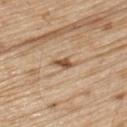<lesion>
<biopsy_status>not biopsied; imaged during a skin examination</biopsy_status>
<site>left upper arm</site>
<image>
  <source>total-body photography crop</source>
  <field_of_view_mm>15</field_of_view_mm>
</image>
<automated_metrics>
  <border_irregularity_0_10>3.5</border_irregularity_0_10>
  <color_variation_0_10>1.5</color_variation_0_10>
  <peripheral_color_asymmetry>0.5</peripheral_color_asymmetry>
  <nevus_likeness_0_100>55</nevus_likeness_0_100>
  <lesion_detection_confidence_0_100>100</lesion_detection_confidence_0_100>
</automated_metrics>
<patient>
  <sex>male</sex>
  <age_approx>70</age_approx>
</patient>
<lesion_size>
  <long_diameter_mm_approx>2.5</long_diameter_mm_approx>
</lesion_size>
</lesion>